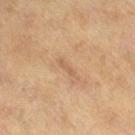notes: total-body-photography surveillance lesion; no biopsy
image-analysis metrics: a lesion color around L≈50 a*≈16 b*≈29 in CIELAB and a normalized border contrast of about 4.5; a border-irregularity rating of about 3/10, a within-lesion color-variation index near 0/10, and peripheral color asymmetry of about 0
image: total-body-photography crop, ~15 mm field of view
subject: female, aged 38 to 42
site: the right thigh